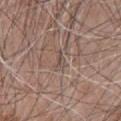Q: Is there a histopathology result?
A: no biopsy performed (imaged during a skin exam)
Q: Lesion location?
A: the chest
Q: Patient demographics?
A: male, about 65 years old
Q: What kind of image is this?
A: 15 mm crop, total-body photography
Q: How was the tile lit?
A: white-light illumination
Q: What is the lesion's diameter?
A: ~1.5 mm (longest diameter)